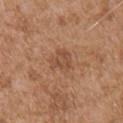Captured during whole-body skin photography for melanoma surveillance; the lesion was not biopsied. The lesion is on the arm. Approximately 3 mm at its widest. Automated image analysis of the tile measured an area of roughly 6.5 mm² and a shape eccentricity near 0.45. The software also gave a lesion color around L≈50 a*≈21 b*≈32 in CIELAB, a lesion–skin lightness drop of about 7, and a lesion-to-skin contrast of about 5.5 (normalized; higher = more distinct). And it measured a nevus-likeness score of about 10/100 and a detector confidence of about 100 out of 100 that the crop contains a lesion. This image is a 15 mm lesion crop taken from a total-body photograph. A male subject, in their mid- to late 70s. Imaged with white-light lighting.